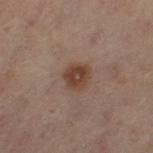biopsy_status: not biopsied; imaged during a skin examination
lighting: cross-polarized
automated_metrics:
  area_mm2_approx: 5.5
  eccentricity: 0.5
  cielab_L: 33
  cielab_a: 16
  cielab_b: 22
  vs_skin_darker_L: 9.0
  vs_skin_contrast_norm: 9.0
  border_irregularity_0_10: 1.5
  color_variation_0_10: 2.5
  lesion_detection_confidence_0_100: 100
patient:
  sex: female
  age_approx: 55
site: left thigh
image:
  source: total-body photography crop
  field_of_view_mm: 15
lesion_size:
  long_diameter_mm_approx: 2.5